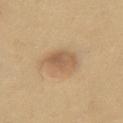The lesion was photographed on a routine skin check and not biopsied; there is no pathology result.
Cropped from a total-body skin-imaging series; the visible field is about 15 mm.
Imaged with cross-polarized lighting.
About 4.5 mm across.
From the chest.
The total-body-photography lesion software estimated a lesion area of about 10 mm², an eccentricity of roughly 0.75, and two-axis asymmetry of about 0.2. The software also gave a border-irregularity index near 2/10, a within-lesion color-variation index near 4/10, and a peripheral color-asymmetry measure near 1.5.
The patient is a male aged around 45.